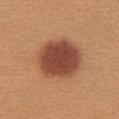Impression: Captured during whole-body skin photography for melanoma surveillance; the lesion was not biopsied. Background: A 15 mm close-up extracted from a 3D total-body photography capture. Imaged with white-light lighting. On the chest. Automated image analysis of the tile measured a lesion color around L≈46 a*≈25 b*≈31 in CIELAB and a lesion–skin lightness drop of about 15. A female subject, approximately 25 years of age. The lesion's longest dimension is about 6 mm.